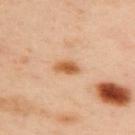{"site": "upper back", "automated_metrics": {"area_mm2_approx": 4.0, "eccentricity": 0.85}, "lesion_size": {"long_diameter_mm_approx": 3.0}, "patient": {"sex": "female", "age_approx": 40}, "lighting": "cross-polarized", "image": {"source": "total-body photography crop", "field_of_view_mm": 15}}